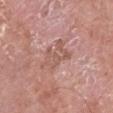Clinical impression: The lesion was photographed on a routine skin check and not biopsied; there is no pathology result. Acquisition and patient details: The subject is a male aged 53 to 57. The tile uses white-light illumination. Automated image analysis of the tile measured roughly 7 lightness units darker than nearby skin. The analysis additionally found a border-irregularity index near 6.5/10 and a color-variation rating of about 3/10. And it measured an automated nevus-likeness rating near 0 out of 100 and a detector confidence of about 100 out of 100 that the crop contains a lesion. The lesion is on the left lower leg. Cropped from a total-body skin-imaging series; the visible field is about 15 mm.